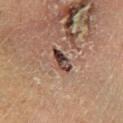workup: total-body-photography surveillance lesion; no biopsy
subject: female, aged 48 to 52
location: the left lower leg
image source: ~15 mm tile from a whole-body skin photo
diameter: about 3.5 mm